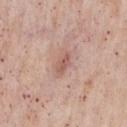This lesion was catalogued during total-body skin photography and was not selected for biopsy. A 15 mm close-up tile from a total-body photography series done for melanoma screening. From the chest. The recorded lesion diameter is about 3 mm. A male patient, in their mid-50s. The lesion-visualizer software estimated an area of roughly 3.5 mm², an outline eccentricity of about 0.85 (0 = round, 1 = elongated), and a symmetry-axis asymmetry near 0.3. It also reported an average lesion color of about L≈57 a*≈22 b*≈25 (CIELAB), a lesion–skin lightness drop of about 10, and a normalized lesion–skin contrast near 6.5.